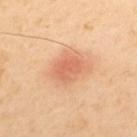workup: no biopsy performed (imaged during a skin exam); imaging modality: ~15 mm tile from a whole-body skin photo; site: the upper back; subject: male, about 55 years old; tile lighting: cross-polarized illumination.